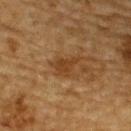Assessment:
Imaged during a routine full-body skin examination; the lesion was not biopsied and no histopathology is available.
Background:
Located on the upper back. A 15 mm close-up tile from a total-body photography series done for melanoma screening. The total-body-photography lesion software estimated a lesion color around L≈35 a*≈18 b*≈33 in CIELAB, roughly 7 lightness units darker than nearby skin, and a normalized lesion–skin contrast near 7. Longest diameter approximately 3.5 mm. The subject is a male in their mid-80s or older.